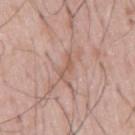* notes — imaged on a skin check; not biopsied
* image — ~15 mm tile from a whole-body skin photo
* image-analysis metrics — a lesion area of about 2.5 mm², an outline eccentricity of about 0.9 (0 = round, 1 = elongated), and a shape-asymmetry score of about 0.75 (0 = symmetric); an average lesion color of about L≈57 a*≈20 b*≈27 (CIELAB) and a lesion-to-skin contrast of about 5.5 (normalized; higher = more distinct); a nevus-likeness score of about 0/100
* illumination — white-light illumination
* diameter — ~3 mm (longest diameter)
* subject — male, aged 53 to 57